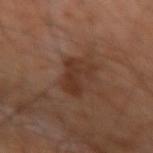– follow-up · total-body-photography surveillance lesion; no biopsy
– anatomic site · the left forearm
– TBP lesion metrics · a lesion area of about 9.5 mm², a shape eccentricity near 0.8, and a symmetry-axis asymmetry near 0.3; border irregularity of about 4 on a 0–10 scale and a peripheral color-asymmetry measure near 1
– imaging modality · ~15 mm crop, total-body skin-cancer survey
– patient · male, aged 63–67